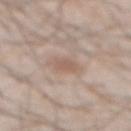Captured during whole-body skin photography for melanoma surveillance; the lesion was not biopsied.
The patient is a male in their mid- to late 60s.
The lesion's longest dimension is about 3 mm.
The lesion is on the abdomen.
This image is a 15 mm lesion crop taken from a total-body photograph.